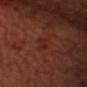follow-up = catalogued during a skin exam; not biopsied
site = the head or neck
lighting = cross-polarized illumination
acquisition = ~15 mm crop, total-body skin-cancer survey
subject = male, aged 63 to 67
diameter = ~2.5 mm (longest diameter)